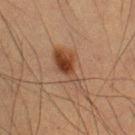biopsy_status: not biopsied; imaged during a skin examination
image:
  source: total-body photography crop
  field_of_view_mm: 15
patient:
  sex: male
  age_approx: 50
site: left upper arm
lighting: cross-polarized
automated_metrics:
  area_mm2_approx: 11.0
  eccentricity: 0.9
  shape_asymmetry: 0.5
  cielab_L: 35
  cielab_a: 17
  cielab_b: 26
  vs_skin_darker_L: 9.0
  vs_skin_contrast_norm: 8.5
lesion_size:
  long_diameter_mm_approx: 6.5